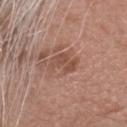{"biopsy_status": "not biopsied; imaged during a skin examination", "site": "head or neck", "image": {"source": "total-body photography crop", "field_of_view_mm": 15}, "patient": {"sex": "male", "age_approx": 75}, "lighting": "white-light", "automated_metrics": {"area_mm2_approx": 6.5}}